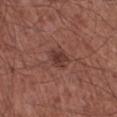Findings:
- automated lesion analysis: an area of roughly 4.5 mm² and two-axis asymmetry of about 0.35; border irregularity of about 3.5 on a 0–10 scale, a within-lesion color-variation index near 3/10, and a peripheral color-asymmetry measure near 1
- tile lighting: white-light illumination
- acquisition: 15 mm crop, total-body photography
- lesion size: about 2.5 mm
- subject: male, about 65 years old
- site: the right lower leg